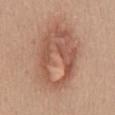biopsy status — catalogued during a skin exam; not biopsied
patient — female, roughly 45 years of age
site — the lower back
image — 15 mm crop, total-body photography